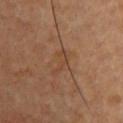biopsy status: catalogued during a skin exam; not biopsied | subject: male, in their mid-40s | anatomic site: the chest | lesion diameter: ~3.5 mm (longest diameter) | image: total-body-photography crop, ~15 mm field of view | tile lighting: cross-polarized.